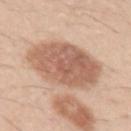notes: no biopsy performed (imaged during a skin exam) | site: the left upper arm | subject: male, approximately 30 years of age | lesion size: about 8 mm | image source: total-body-photography crop, ~15 mm field of view.